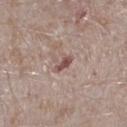Clinical impression: Recorded during total-body skin imaging; not selected for excision or biopsy. Clinical summary: Approximately 2.5 mm at its widest. A male patient, in their mid- to late 40s. The tile uses white-light illumination. From the left lower leg. A lesion tile, about 15 mm wide, cut from a 3D total-body photograph.